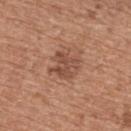Assessment: Part of a total-body skin-imaging series; this lesion was reviewed on a skin check and was not flagged for biopsy. Clinical summary: The subject is a female about 60 years old. The lesion is on the upper back. A 15 mm crop from a total-body photograph taken for skin-cancer surveillance. Measured at roughly 3.5 mm in maximum diameter. The tile uses white-light illumination.